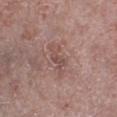biopsy status: imaged on a skin check; not biopsied | illumination: white-light illumination | location: the left lower leg | TBP lesion metrics: a lesion area of about 6 mm², an eccentricity of roughly 0.9, and a symmetry-axis asymmetry near 0.4; a mean CIELAB color near L≈50 a*≈19 b*≈22 and roughly 7 lightness units darker than nearby skin; a border-irregularity index near 5/10 and a within-lesion color-variation index near 2.5/10 | size: about 4 mm | image: 15 mm crop, total-body photography | subject: male, roughly 65 years of age.